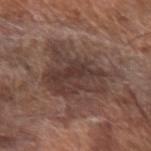Q: Was this lesion biopsied?
A: total-body-photography surveillance lesion; no biopsy
Q: What is the imaging modality?
A: ~15 mm crop, total-body skin-cancer survey
Q: Automated lesion metrics?
A: an outline eccentricity of about 0.6 (0 = round, 1 = elongated); a nevus-likeness score of about 5/100 and a lesion-detection confidence of about 90/100
Q: Where on the body is the lesion?
A: the left forearm
Q: What lighting was used for the tile?
A: white-light
Q: What are the patient's age and sex?
A: male, aged 68–72